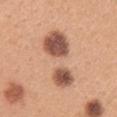Clinical impression:
No biopsy was performed on this lesion — it was imaged during a full skin examination and was not determined to be concerning.
Context:
Captured under white-light illumination. A female patient, about 30 years old. On the right upper arm. Automated image analysis of the tile measured a lesion area of about 27 mm², an outline eccentricity of about 0.85 (0 = round, 1 = elongated), and a symmetry-axis asymmetry near 0.3. And it measured a within-lesion color-variation index near 10/10 and a peripheral color-asymmetry measure near 4. A roughly 15 mm field-of-view crop from a total-body skin photograph. About 8 mm across.